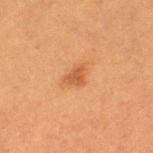Q: What is the anatomic site?
A: the left thigh
Q: What is the lesion's diameter?
A: ≈2.5 mm
Q: Automated lesion metrics?
A: an outline eccentricity of about 0.65 (0 = round, 1 = elongated); border irregularity of about 3.5 on a 0–10 scale, a within-lesion color-variation index near 1/10, and radial color variation of about 0.5; a nevus-likeness score of about 70/100 and a detector confidence of about 100 out of 100 that the crop contains a lesion
Q: Patient demographics?
A: female, approximately 40 years of age
Q: What is the imaging modality?
A: 15 mm crop, total-body photography
Q: Illumination type?
A: cross-polarized